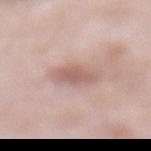This lesion was catalogued during total-body skin photography and was not selected for biopsy.